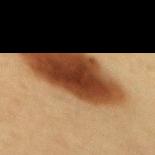{
  "biopsy_status": "not biopsied; imaged during a skin examination",
  "lighting": "cross-polarized",
  "patient": {
    "sex": "female",
    "age_approx": 40
  },
  "site": "mid back",
  "lesion_size": {
    "long_diameter_mm_approx": 10.5
  },
  "image": {
    "source": "total-body photography crop",
    "field_of_view_mm": 15
  }
}